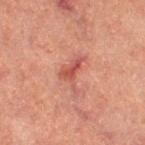The lesion was tiled from a total-body skin photograph and was not biopsied.
The tile uses cross-polarized illumination.
This image is a 15 mm lesion crop taken from a total-body photograph.
About 4.5 mm across.
The lesion is located on the right thigh.
The subject is a female aged 58–62.